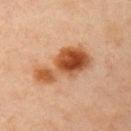Context:
A female patient aged 43–47. The recorded lesion diameter is about 6.5 mm. This image is a 15 mm lesion crop taken from a total-body photograph. On the left upper arm.